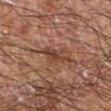The lesion-visualizer software estimated a border-irregularity rating of about 3.5/10 and radial color variation of about 1. This is a cross-polarized tile. From the right forearm. The patient is a male aged around 70. Approximately 4 mm at its widest. A 15 mm close-up tile from a total-body photography series done for melanoma screening.